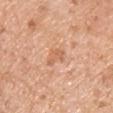Notes:
- workup: no biopsy performed (imaged during a skin exam)
- site: the chest
- subject: male, aged 58 to 62
- illumination: white-light illumination
- imaging modality: total-body-photography crop, ~15 mm field of view
- lesion size: ~3 mm (longest diameter)